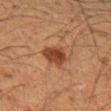biopsy status: imaged on a skin check; not biopsied | subject: male, aged approximately 50 | size: ~3.5 mm (longest diameter) | image source: total-body-photography crop, ~15 mm field of view | anatomic site: the left upper arm.